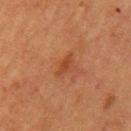Background: On the left upper arm. A female patient about 55 years old. The lesion's longest dimension is about 2.5 mm. A lesion tile, about 15 mm wide, cut from a 3D total-body photograph.